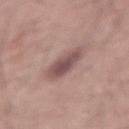– follow-up — no biopsy performed (imaged during a skin exam)
– automated metrics — a lesion–skin lightness drop of about 12 and a lesion-to-skin contrast of about 8.5 (normalized; higher = more distinct); a border-irregularity index near 1.5/10, a within-lesion color-variation index near 3.5/10, and a peripheral color-asymmetry measure near 1; an automated nevus-likeness rating near 40 out of 100 and a lesion-detection confidence of about 100/100
– body site — the lower back
– image source — ~15 mm crop, total-body skin-cancer survey
– lesion diameter — ~4.5 mm (longest diameter)
– lighting — white-light illumination
– subject — male, roughly 20 years of age